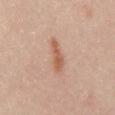Imaged during a routine full-body skin examination; the lesion was not biopsied and no histopathology is available. A male patient, aged 38–42. Cropped from a whole-body photographic skin survey; the tile spans about 15 mm. On the front of the torso. Captured under cross-polarized illumination.